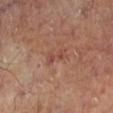The lesion was photographed on a routine skin check and not biopsied; there is no pathology result. A 15 mm close-up tile from a total-body photography series done for melanoma screening. The lesion is located on the left lower leg.